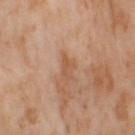Q: Is there a histopathology result?
A: catalogued during a skin exam; not biopsied
Q: How was the tile lit?
A: cross-polarized illumination
Q: Patient demographics?
A: female, in their mid-50s
Q: What is the lesion's diameter?
A: ~3 mm (longest diameter)
Q: Where on the body is the lesion?
A: the right thigh
Q: What kind of image is this?
A: ~15 mm crop, total-body skin-cancer survey